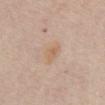biopsy_status: not biopsied; imaged during a skin examination